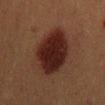  biopsy_status: not biopsied; imaged during a skin examination
  lighting: cross-polarized
  site: mid back
  image:
    source: total-body photography crop
    field_of_view_mm: 15
  patient:
    sex: male
    age_approx: 40